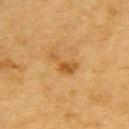<lesion>
<biopsy_status>not biopsied; imaged during a skin examination</biopsy_status>
<lighting>cross-polarized</lighting>
<site>upper back</site>
<image>
  <source>total-body photography crop</source>
  <field_of_view_mm>15</field_of_view_mm>
</image>
<lesion_size>
  <long_diameter_mm_approx>4.0</long_diameter_mm_approx>
</lesion_size>
<automated_metrics>
  <eccentricity>0.9</eccentricity>
  <shape_asymmetry>0.55</shape_asymmetry>
  <cielab_L>48</cielab_L>
  <cielab_a>20</cielab_a>
  <cielab_b>41</cielab_b>
  <vs_skin_darker_L>8.0</vs_skin_darker_L>
  <vs_skin_contrast_norm>7.0</vs_skin_contrast_norm>
</automated_metrics>
<patient>
  <sex>male</sex>
  <age_approx>85+</age_approx>
</patient>
</lesion>